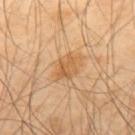<case>
<biopsy_status>not biopsied; imaged during a skin examination</biopsy_status>
<image>
  <source>total-body photography crop</source>
  <field_of_view_mm>15</field_of_view_mm>
</image>
<patient>
  <sex>male</sex>
  <age_approx>40</age_approx>
</patient>
<automated_metrics>
  <area_mm2_approx>7.5</area_mm2_approx>
  <eccentricity>0.7</eccentricity>
  <cielab_L>61</cielab_L>
  <cielab_a>20</cielab_a>
  <cielab_b>41</cielab_b>
</automated_metrics>
<site>upper back</site>
<lesion_size>
  <long_diameter_mm_approx>3.5</long_diameter_mm_approx>
</lesion_size>
</case>